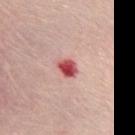Case summary:
– follow-up — no biopsy performed (imaged during a skin exam)
– location — the chest
– lighting — white-light illumination
– lesion size — ~2.5 mm (longest diameter)
– image source — 15 mm crop, total-body photography
– patient — female, in their mid- to late 60s